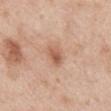lighting: white-light
imaging modality: 15 mm crop, total-body photography
TBP lesion metrics: a footprint of about 4 mm², a shape eccentricity near 0.65, and a symmetry-axis asymmetry near 0.25; an average lesion color of about L≈58 a*≈23 b*≈31 (CIELAB), about 11 CIELAB-L* units darker than the surrounding skin, and a lesion-to-skin contrast of about 7 (normalized; higher = more distinct); a border-irregularity index near 2.5/10, a color-variation rating of about 3.5/10, and radial color variation of about 1.5
patient: female, aged 38 to 42
location: the back